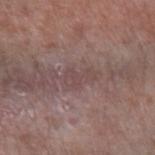Q: What is the imaging modality?
A: ~15 mm tile from a whole-body skin photo
Q: What lighting was used for the tile?
A: white-light illumination
Q: How large is the lesion?
A: ~3.5 mm (longest diameter)
Q: Who is the patient?
A: female, in their mid-60s
Q: Where on the body is the lesion?
A: the left forearm